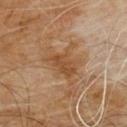Recorded during total-body skin imaging; not selected for excision or biopsy. Measured at roughly 6 mm in maximum diameter. Imaged with cross-polarized lighting. On the chest. A 15 mm crop from a total-body photograph taken for skin-cancer surveillance. A male patient in their 60s.